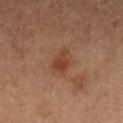Findings:
* notes: catalogued during a skin exam; not biopsied
* patient: female, in their mid-40s
* image: total-body-photography crop, ~15 mm field of view
* anatomic site: the right upper arm